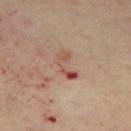Q: What lighting was used for the tile?
A: cross-polarized illumination
Q: Patient demographics?
A: male, aged around 55
Q: Lesion size?
A: ~3.5 mm (longest diameter)
Q: Automated lesion metrics?
A: an area of roughly 5 mm² and two-axis asymmetry of about 0.45; an average lesion color of about L≈53 a*≈23 b*≈29 (CIELAB) and about 10 CIELAB-L* units darker than the surrounding skin; a border-irregularity rating of about 6.5/10, a within-lesion color-variation index near 6/10, and radial color variation of about 0.5
Q: Where on the body is the lesion?
A: the left thigh
Q: How was this image acquired?
A: ~15 mm crop, total-body skin-cancer survey